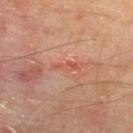| feature | finding |
|---|---|
| biopsy status | total-body-photography surveillance lesion; no biopsy |
| site | the upper back |
| patient | male, aged around 65 |
| image | ~15 mm tile from a whole-body skin photo |
| size | ~3 mm (longest diameter) |
| lighting | cross-polarized illumination |
| automated lesion analysis | a lesion color around L≈54 a*≈30 b*≈33 in CIELAB and roughly 7 lightness units darker than nearby skin; a classifier nevus-likeness of about 0/100 and a detector confidence of about 75 out of 100 that the crop contains a lesion |